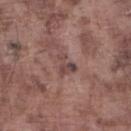notes: no biopsy performed (imaged during a skin exam)
image source: ~15 mm tile from a whole-body skin photo
image-analysis metrics: a mean CIELAB color near L≈44 a*≈18 b*≈20, about 9 CIELAB-L* units darker than the surrounding skin, and a lesion-to-skin contrast of about 7 (normalized; higher = more distinct); a classifier nevus-likeness of about 0/100 and a detector confidence of about 85 out of 100 that the crop contains a lesion
tile lighting: white-light illumination
subject: male, in their mid-70s
size: about 2.5 mm
site: the left lower leg